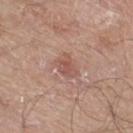Q: Is there a histopathology result?
A: no biopsy performed (imaged during a skin exam)
Q: Patient demographics?
A: male, aged 78 to 82
Q: What did automated image analysis measure?
A: border irregularity of about 3 on a 0–10 scale
Q: What is the anatomic site?
A: the right thigh
Q: What is the lesion's diameter?
A: ~3 mm (longest diameter)
Q: How was this image acquired?
A: ~15 mm crop, total-body skin-cancer survey